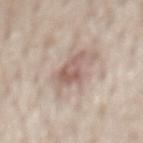Q: Was a biopsy performed?
A: catalogued during a skin exam; not biopsied
Q: Patient demographics?
A: male, roughly 75 years of age
Q: What is the imaging modality?
A: total-body-photography crop, ~15 mm field of view
Q: Where on the body is the lesion?
A: the mid back
Q: Illumination type?
A: white-light illumination